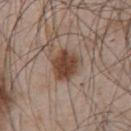Q: Was a biopsy performed?
A: catalogued during a skin exam; not biopsied
Q: How was this image acquired?
A: 15 mm crop, total-body photography
Q: Lesion size?
A: ~4 mm (longest diameter)
Q: What are the patient's age and sex?
A: male, aged 43 to 47
Q: Lesion location?
A: the chest
Q: What did automated image analysis measure?
A: a lesion–skin lightness drop of about 13 and a lesion-to-skin contrast of about 10.5 (normalized; higher = more distinct); a detector confidence of about 100 out of 100 that the crop contains a lesion
Q: How was the tile lit?
A: white-light illumination